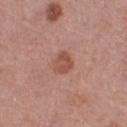Impression:
The lesion was tiled from a total-body skin photograph and was not biopsied.
Acquisition and patient details:
The tile uses white-light illumination. The lesion's longest dimension is about 2.5 mm. Cropped from a whole-body photographic skin survey; the tile spans about 15 mm. Automated image analysis of the tile measured an area of roughly 5 mm². It also reported a lesion color around L≈51 a*≈24 b*≈27 in CIELAB, roughly 9 lightness units darker than nearby skin, and a lesion-to-skin contrast of about 7 (normalized; higher = more distinct). It also reported a lesion-detection confidence of about 100/100. Located on the leg. A female subject aged approximately 65.